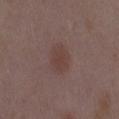{"automated_metrics": {"area_mm2_approx": 7.5, "eccentricity": 0.75, "shape_asymmetry": 0.2, "cielab_L": 40, "cielab_a": 17, "cielab_b": 20, "vs_skin_darker_L": 6.0, "color_variation_0_10": 2.0, "peripheral_color_asymmetry": 0.5}, "patient": {"sex": "female", "age_approx": 35}, "lighting": "white-light", "image": {"source": "total-body photography crop", "field_of_view_mm": 15}, "lesion_size": {"long_diameter_mm_approx": 3.5}, "site": "lower back"}